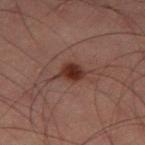follow-up: no biopsy performed (imaged during a skin exam); illumination: cross-polarized; body site: the left thigh; patient: male, aged 58–62; lesion size: ≈2.5 mm; image source: ~15 mm crop, total-body skin-cancer survey.